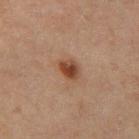Q: Was this lesion biopsied?
A: total-body-photography surveillance lesion; no biopsy
Q: Patient demographics?
A: male, about 65 years old
Q: How was this image acquired?
A: ~15 mm tile from a whole-body skin photo
Q: Where on the body is the lesion?
A: the mid back
Q: What did automated image analysis measure?
A: a border-irregularity rating of about 2.5/10, a within-lesion color-variation index near 4/10, and peripheral color asymmetry of about 1; a classifier nevus-likeness of about 100/100 and lesion-presence confidence of about 100/100